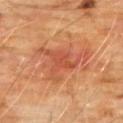<record>
<biopsy_status>not biopsied; imaged during a skin examination</biopsy_status>
<site>front of the torso</site>
<lesion_size>
  <long_diameter_mm_approx>7.0</long_diameter_mm_approx>
</lesion_size>
<image>
  <source>total-body photography crop</source>
  <field_of_view_mm>15</field_of_view_mm>
</image>
<patient>
  <sex>male</sex>
  <age_approx>60</age_approx>
</patient>
<lighting>cross-polarized</lighting>
</record>